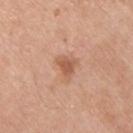{"biopsy_status": "not biopsied; imaged during a skin examination", "automated_metrics": {"area_mm2_approx": 4.0, "eccentricity": 0.6, "shape_asymmetry": 0.4, "border_irregularity_0_10": 3.5, "color_variation_0_10": 1.5, "peripheral_color_asymmetry": 0.5}, "lesion_size": {"long_diameter_mm_approx": 2.5}, "patient": {"sex": "male", "age_approx": 75}, "image": {"source": "total-body photography crop", "field_of_view_mm": 15}, "site": "back", "lighting": "white-light"}